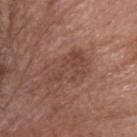Captured during whole-body skin photography for melanoma surveillance; the lesion was not biopsied. About 5 mm across. From the head or neck. The subject is a male roughly 50 years of age. Automated image analysis of the tile measured an area of roughly 10 mm² and a symmetry-axis asymmetry near 0.5. The software also gave a color-variation rating of about 3/10 and a peripheral color-asymmetry measure near 1. A close-up tile cropped from a whole-body skin photograph, about 15 mm across. Captured under white-light illumination.